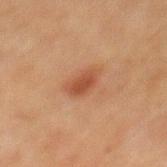notes — catalogued during a skin exam; not biopsied
diameter — about 3.5 mm
patient — male, roughly 50 years of age
tile lighting — cross-polarized
image source — ~15 mm crop, total-body skin-cancer survey
location — the mid back